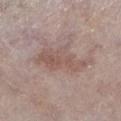follow-up: imaged on a skin check; not biopsied
anatomic site: the left lower leg
image source: ~15 mm crop, total-body skin-cancer survey
subject: male, aged 78–82
image-analysis metrics: a footprint of about 13 mm², a shape eccentricity near 0.9, and a shape-asymmetry score of about 0.4 (0 = symmetric); a nevus-likeness score of about 0/100 and a lesion-detection confidence of about 95/100